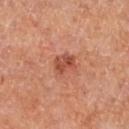Imaged during a routine full-body skin examination; the lesion was not biopsied and no histopathology is available. Located on the left lower leg. A 15 mm close-up tile from a total-body photography series done for melanoma screening. Approximately 3 mm at its widest. The patient is female. The tile uses cross-polarized illumination.